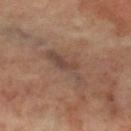| feature | finding |
|---|---|
| size | ≈5.5 mm |
| TBP lesion metrics | an area of roughly 8.5 mm² and a shape-asymmetry score of about 0.4 (0 = symmetric); border irregularity of about 6 on a 0–10 scale and radial color variation of about 0.5; an automated nevus-likeness rating near 0 out of 100 and lesion-presence confidence of about 55/100 |
| image | ~15 mm crop, total-body skin-cancer survey |
| site | the left leg |
| tile lighting | cross-polarized |
| patient | female, roughly 65 years of age |